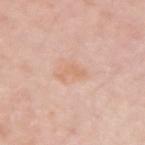This lesion was catalogued during total-body skin photography and was not selected for biopsy.
The subject is a female approximately 40 years of age.
A 15 mm close-up tile from a total-body photography series done for melanoma screening.
The lesion is on the arm.
Automated image analysis of the tile measured internal color variation of about 0 on a 0–10 scale and a peripheral color-asymmetry measure near 0. And it measured an automated nevus-likeness rating near 0 out of 100 and lesion-presence confidence of about 100/100.